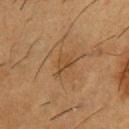Clinical impression: Recorded during total-body skin imaging; not selected for excision or biopsy. Context: A close-up tile cropped from a whole-body skin photograph, about 15 mm across. The lesion is on the back. The patient is a male aged approximately 55. The tile uses cross-polarized illumination.